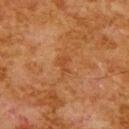Q: Was a biopsy performed?
A: imaged on a skin check; not biopsied
Q: Lesion location?
A: the upper back
Q: Lesion size?
A: ~2.5 mm (longest diameter)
Q: How was this image acquired?
A: ~15 mm crop, total-body skin-cancer survey
Q: Illumination type?
A: cross-polarized
Q: What are the patient's age and sex?
A: male, aged approximately 80